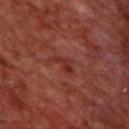| field | value |
|---|---|
| follow-up | no biopsy performed (imaged during a skin exam) |
| acquisition | ~15 mm crop, total-body skin-cancer survey |
| anatomic site | the upper back |
| patient | male, roughly 70 years of age |
| lesion size | about 3.5 mm |